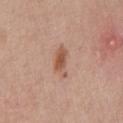Clinical impression: The lesion was tiled from a total-body skin photograph and was not biopsied. Context: The lesion's longest dimension is about 4 mm. A lesion tile, about 15 mm wide, cut from a 3D total-body photograph. Captured under white-light illumination. The subject is a male about 55 years old. From the chest.